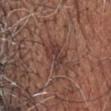- workup · catalogued during a skin exam; not biopsied
- location · the head or neck
- subject · male, aged around 65
- automated metrics · a footprint of about 6.5 mm² and two-axis asymmetry of about 0.35; a lesion color around L≈37 a*≈19 b*≈22 in CIELAB, a lesion–skin lightness drop of about 8, and a normalized border contrast of about 7.5; a nevus-likeness score of about 10/100 and a detector confidence of about 95 out of 100 that the crop contains a lesion
- imaging modality · total-body-photography crop, ~15 mm field of view
- lesion diameter · about 4 mm
- tile lighting · white-light illumination